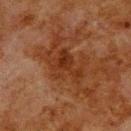<case>
  <biopsy_status>not biopsied; imaged during a skin examination</biopsy_status>
  <lesion_size>
    <long_diameter_mm_approx>4.5</long_diameter_mm_approx>
  </lesion_size>
  <lighting>cross-polarized</lighting>
  <patient>
    <sex>male</sex>
    <age_approx>80</age_approx>
  </patient>
  <image>
    <source>total-body photography crop</source>
    <field_of_view_mm>15</field_of_view_mm>
  </image>
  <site>upper back</site>
</case>